Cropped from a total-body skin-imaging series; the visible field is about 15 mm, located on the back, a female patient aged 38 to 42 — 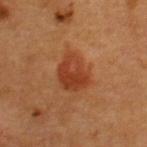Biopsy histopathology demonstrated a melanoma in situ.